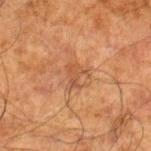This lesion was catalogued during total-body skin photography and was not selected for biopsy.
A 15 mm crop from a total-body photograph taken for skin-cancer surveillance.
Located on the right lower leg.
Longest diameter approximately 3 mm.
Captured under cross-polarized illumination.
A male patient, aged around 70.